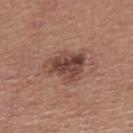A male patient aged 38 to 42. A roughly 15 mm field-of-view crop from a total-body skin photograph. The tile uses white-light illumination. Located on the upper back. Approximately 4.5 mm at its widest.